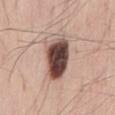Impression: Captured during whole-body skin photography for melanoma surveillance; the lesion was not biopsied. Clinical summary: The lesion's longest dimension is about 5 mm. A 15 mm close-up tile from a total-body photography series done for melanoma screening. An algorithmic analysis of the crop reported a footprint of about 16 mm², an eccentricity of roughly 0.7, and a symmetry-axis asymmetry near 0.2. The software also gave an average lesion color of about L≈46 a*≈18 b*≈22 (CIELAB), a lesion–skin lightness drop of about 23, and a normalized border contrast of about 15.5. From the lower back. The tile uses white-light illumination. The patient is a male roughly 30 years of age.